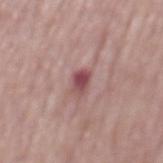Case summary:
• follow-up: total-body-photography surveillance lesion; no biopsy
• tile lighting: white-light
• location: the mid back
• lesion size: ≈2.5 mm
• image-analysis metrics: a classifier nevus-likeness of about 10/100
• subject: male, about 65 years old
• image: ~15 mm tile from a whole-body skin photo